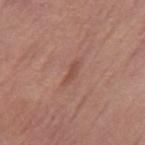follow-up = total-body-photography surveillance lesion; no biopsy
illumination = white-light illumination
lesion diameter = about 2.5 mm
automated metrics = an average lesion color of about L≈49 a*≈23 b*≈27 (CIELAB) and a normalized lesion–skin contrast near 6
image = total-body-photography crop, ~15 mm field of view
anatomic site = the left leg
subject = female, aged around 65